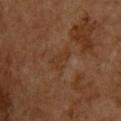Recorded during total-body skin imaging; not selected for excision or biopsy.